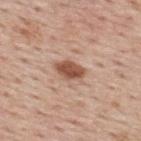| key | value |
|---|---|
| follow-up | no biopsy performed (imaged during a skin exam) |
| illumination | white-light |
| site | the back |
| TBP lesion metrics | a color-variation rating of about 2.5/10 and radial color variation of about 1; a nevus-likeness score of about 95/100 and lesion-presence confidence of about 100/100 |
| subject | male, aged 73 to 77 |
| acquisition | 15 mm crop, total-body photography |